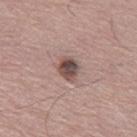{
  "biopsy_status": "not biopsied; imaged during a skin examination",
  "patient": {
    "sex": "male",
    "age_approx": 55
  },
  "lesion_size": {
    "long_diameter_mm_approx": 2.5
  },
  "image": {
    "source": "total-body photography crop",
    "field_of_view_mm": 15
  },
  "automated_metrics": {
    "area_mm2_approx": 5.0,
    "eccentricity": 0.6,
    "shape_asymmetry": 0.15
  },
  "lighting": "white-light"
}